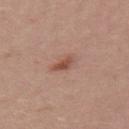Q: Was a biopsy performed?
A: total-body-photography surveillance lesion; no biopsy
Q: Patient demographics?
A: female, aged approximately 25
Q: What is the lesion's diameter?
A: ~3 mm (longest diameter)
Q: How was this image acquired?
A: ~15 mm crop, total-body skin-cancer survey
Q: Where on the body is the lesion?
A: the upper back
Q: Illumination type?
A: white-light